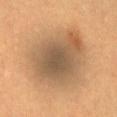Assessment:
The lesion was tiled from a total-body skin photograph and was not biopsied.
Background:
The patient is a female aged around 30. Longest diameter approximately 7.5 mm. Captured under cross-polarized illumination. The lesion is on the front of the torso. Cropped from a total-body skin-imaging series; the visible field is about 15 mm.